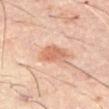Findings:
• location — the abdomen
• image source — ~15 mm tile from a whole-body skin photo
• image-analysis metrics — an area of roughly 8 mm², an outline eccentricity of about 0.65 (0 = round, 1 = elongated), and a symmetry-axis asymmetry near 0.2; a lesion color around L≈60 a*≈22 b*≈31 in CIELAB and a normalized lesion–skin contrast near 6.5
• patient — male, aged 58–62
• lighting — cross-polarized illumination